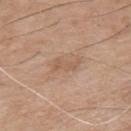biopsy_status: not biopsied; imaged during a skin examination
patient:
  sex: male
  age_approx: 75
site: mid back
automated_metrics:
  lesion_detection_confidence_0_100: 100
lesion_size:
  long_diameter_mm_approx: 3.0
image:
  source: total-body photography crop
  field_of_view_mm: 15
lighting: white-light